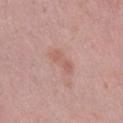Recorded during total-body skin imaging; not selected for excision or biopsy. A female subject aged 38 to 42. An algorithmic analysis of the crop reported a shape eccentricity near 0.9 and a shape-asymmetry score of about 0.3 (0 = symmetric). From the leg. The lesion's longest dimension is about 3.5 mm. A 15 mm crop from a total-body photograph taken for skin-cancer surveillance. The tile uses white-light illumination.